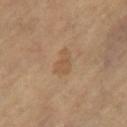Imaged with cross-polarized lighting. The lesion-visualizer software estimated a lesion color around L≈53 a*≈16 b*≈33 in CIELAB and roughly 6 lightness units darker than nearby skin. It also reported a within-lesion color-variation index near 1.5/10 and radial color variation of about 0.5. A female patient, aged 63 to 67. Cropped from a total-body skin-imaging series; the visible field is about 15 mm. The lesion is located on the right thigh. Longest diameter approximately 3.5 mm.